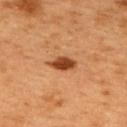| key | value |
|---|---|
| notes | imaged on a skin check; not biopsied |
| site | the upper back |
| subject | male, aged 48–52 |
| image source | 15 mm crop, total-body photography |
| illumination | cross-polarized |
| lesion size | ~3.5 mm (longest diameter) |